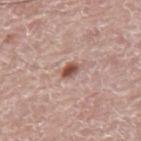• notes — no biopsy performed (imaged during a skin exam)
• site — the mid back
• size — about 2.5 mm
• patient — male, aged approximately 75
• image-analysis metrics — an outline eccentricity of about 0.8 (0 = round, 1 = elongated) and a shape-asymmetry score of about 0.25 (0 = symmetric); an automated nevus-likeness rating near 95 out of 100 and a lesion-detection confidence of about 100/100
• imaging modality — ~15 mm tile from a whole-body skin photo